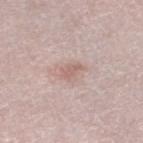Case summary:
• notes — no biopsy performed (imaged during a skin exam)
• automated metrics — a footprint of about 3.5 mm², an outline eccentricity of about 0.75 (0 = round, 1 = elongated), and two-axis asymmetry of about 0.3; border irregularity of about 3 on a 0–10 scale; a lesion-detection confidence of about 100/100
• subject — female, in their mid-50s
• diameter — ~2.5 mm (longest diameter)
• body site — the left thigh
• tile lighting — white-light illumination
• image source — total-body-photography crop, ~15 mm field of view